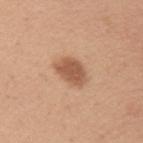| field | value |
|---|---|
| biopsy status | total-body-photography surveillance lesion; no biopsy |
| illumination | white-light illumination |
| lesion diameter | about 3.5 mm |
| site | the arm |
| acquisition | total-body-photography crop, ~15 mm field of view |
| subject | female, roughly 30 years of age |